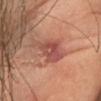workup = catalogued during a skin exam; not biopsied
lesion size = ≈5 mm
patient = female, aged 58 to 62
acquisition = ~15 mm tile from a whole-body skin photo
body site = the head or neck